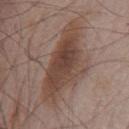Q: Was this lesion biopsied?
A: imaged on a skin check; not biopsied
Q: What is the imaging modality?
A: 15 mm crop, total-body photography
Q: Lesion location?
A: the chest
Q: How large is the lesion?
A: about 9.5 mm
Q: What did automated image analysis measure?
A: a classifier nevus-likeness of about 85/100 and a lesion-detection confidence of about 100/100
Q: Patient demographics?
A: male, aged 63 to 67
Q: Illumination type?
A: white-light illumination